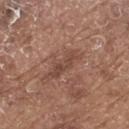Assessment: Imaged during a routine full-body skin examination; the lesion was not biopsied and no histopathology is available. Context: A region of skin cropped from a whole-body photographic capture, roughly 15 mm wide. The tile uses white-light illumination. The lesion's longest dimension is about 4.5 mm. On the upper back. A male subject, aged 78–82. Automated tile analysis of the lesion measured a footprint of about 6 mm², an outline eccentricity of about 0.9 (0 = round, 1 = elongated), and a symmetry-axis asymmetry near 0.4. And it measured a lesion color around L≈45 a*≈20 b*≈26 in CIELAB, about 8 CIELAB-L* units darker than the surrounding skin, and a normalized border contrast of about 6. The software also gave a border-irregularity rating of about 5/10, internal color variation of about 2.5 on a 0–10 scale, and a peripheral color-asymmetry measure near 1. It also reported a nevus-likeness score of about 0/100.